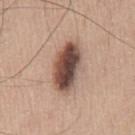  biopsy_status: not biopsied; imaged during a skin examination
  automated_metrics:
    area_mm2_approx: 16.0
    eccentricity: 0.9
    shape_asymmetry: 0.15
    cielab_L: 48
    cielab_a: 18
    cielab_b: 24
    vs_skin_contrast_norm: 13.0
    peripheral_color_asymmetry: 3.0
    nevus_likeness_0_100: 100
    lesion_detection_confidence_0_100: 100
  image:
    source: total-body photography crop
    field_of_view_mm: 15
  site: chest
  lighting: white-light
  patient:
    sex: male
    age_approx: 70